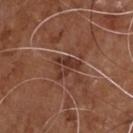| field | value |
|---|---|
| patient | male, approximately 70 years of age |
| image | ~15 mm tile from a whole-body skin photo |
| site | the chest |
| diameter | ~3.5 mm (longest diameter) |
| automated metrics | a border-irregularity index near 5/10, a color-variation rating of about 5/10, and radial color variation of about 2; an automated nevus-likeness rating near 0 out of 100 |